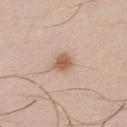The tile uses white-light illumination. The subject is a male aged around 40. A 15 mm close-up extracted from a 3D total-body photography capture. The lesion's longest dimension is about 2.5 mm. The total-body-photography lesion software estimated a lesion area of about 4.5 mm² and a shape-asymmetry score of about 0.25 (0 = symmetric). The software also gave a lesion color around L≈59 a*≈19 b*≈32 in CIELAB and roughly 12 lightness units darker than nearby skin. The analysis additionally found border irregularity of about 2.5 on a 0–10 scale, a within-lesion color-variation index near 2/10, and peripheral color asymmetry of about 0.5. The analysis additionally found an automated nevus-likeness rating near 95 out of 100 and a lesion-detection confidence of about 100/100. On the chest.